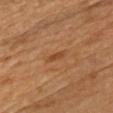workup: no biopsy performed (imaged during a skin exam)
patient: male, aged 68–72
site: the chest
acquisition: ~15 mm crop, total-body skin-cancer survey
lesion diameter: ~3 mm (longest diameter)
tile lighting: cross-polarized illumination